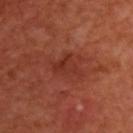| field | value |
|---|---|
| notes | no biopsy performed (imaged during a skin exam) |
| size | ~4 mm (longest diameter) |
| location | the upper back |
| image-analysis metrics | an average lesion color of about L≈34 a*≈29 b*≈29 (CIELAB), roughly 6 lightness units darker than nearby skin, and a normalized border contrast of about 5.5 |
| illumination | cross-polarized illumination |
| patient | male, aged 48–52 |
| image | ~15 mm crop, total-body skin-cancer survey |